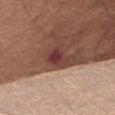Q: Is there a histopathology result?
A: total-body-photography surveillance lesion; no biopsy
Q: How was this image acquired?
A: ~15 mm tile from a whole-body skin photo
Q: Who is the patient?
A: female, in their mid- to late 60s
Q: What is the anatomic site?
A: the right thigh
Q: Illumination type?
A: white-light